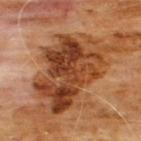Case summary:
* biopsy status — catalogued during a skin exam; not biopsied
* site — the chest
* acquisition — ~15 mm tile from a whole-body skin photo
* patient — male, aged 58–62